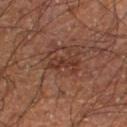{"biopsy_status": "not biopsied; imaged during a skin examination", "lighting": "cross-polarized", "lesion_size": {"long_diameter_mm_approx": 3.5}, "image": {"source": "total-body photography crop", "field_of_view_mm": 15}, "site": "right lower leg", "patient": {"sex": "male", "age_approx": 60}}